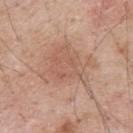Captured during whole-body skin photography for melanoma surveillance; the lesion was not biopsied.